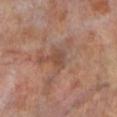Q: Is there a histopathology result?
A: imaged on a skin check; not biopsied
Q: Who is the patient?
A: male, roughly 70 years of age
Q: What is the lesion's diameter?
A: about 2.5 mm
Q: Where on the body is the lesion?
A: the left lower leg
Q: How was this image acquired?
A: 15 mm crop, total-body photography
Q: Illumination type?
A: cross-polarized